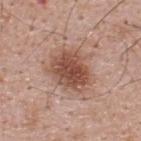workup=imaged on a skin check; not biopsied | acquisition=total-body-photography crop, ~15 mm field of view | illumination=white-light | site=the upper back | automated metrics=roughly 13 lightness units darker than nearby skin; a border-irregularity rating of about 1.5/10, internal color variation of about 5 on a 0–10 scale, and radial color variation of about 1.5 | subject=male, approximately 40 years of age | lesion diameter=about 5 mm.